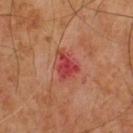Assessment:
The lesion was photographed on a routine skin check and not biopsied; there is no pathology result.
Clinical summary:
A male subject, aged around 65. The tile uses cross-polarized illumination. Cropped from a total-body skin-imaging series; the visible field is about 15 mm. Approximately 3.5 mm at its widest.